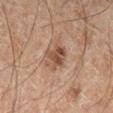<case>
<biopsy_status>not biopsied; imaged during a skin examination</biopsy_status>
<site>left lower leg</site>
<patient>
  <sex>male</sex>
  <age_approx>45</age_approx>
</patient>
<image>
  <source>total-body photography crop</source>
  <field_of_view_mm>15</field_of_view_mm>
</image>
<automated_metrics>
  <area_mm2_approx>7.5</area_mm2_approx>
  <eccentricity>0.4</eccentricity>
  <shape_asymmetry>0.2</shape_asymmetry>
  <cielab_L>40</cielab_L>
  <cielab_a>16</cielab_a>
  <cielab_b>24</cielab_b>
  <vs_skin_contrast_norm>7.5</vs_skin_contrast_norm>
</automated_metrics>
<lesion_size>
  <long_diameter_mm_approx>3.5</long_diameter_mm_approx>
</lesion_size>
<lighting>cross-polarized</lighting>
</case>